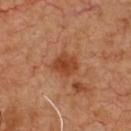The lesion was tiled from a total-body skin photograph and was not biopsied.
The patient is a male approximately 50 years of age.
The lesion is located on the chest.
Cropped from a whole-body photographic skin survey; the tile spans about 15 mm.